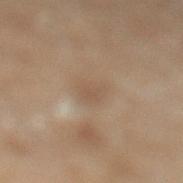Impression: Imaged during a routine full-body skin examination; the lesion was not biopsied and no histopathology is available. Background: Automated tile analysis of the lesion measured an eccentricity of roughly 0.7 and a shape-asymmetry score of about 0.3 (0 = symmetric). The software also gave a mean CIELAB color near L≈39 a*≈11 b*≈23, about 5 CIELAB-L* units darker than the surrounding skin, and a normalized border contrast of about 4.5. It also reported border irregularity of about 3 on a 0–10 scale, a within-lesion color-variation index near 1.5/10, and peripheral color asymmetry of about 0.5. A 15 mm close-up extracted from a 3D total-body photography capture. About 3 mm across. A male subject aged 68 to 72. The tile uses cross-polarized illumination. The lesion is located on the left lower leg.